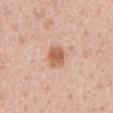Assessment:
Recorded during total-body skin imaging; not selected for excision or biopsy.
Background:
The lesion is on the abdomen. The tile uses white-light illumination. This image is a 15 mm lesion crop taken from a total-body photograph. The recorded lesion diameter is about 3 mm. A male patient, aged approximately 55. An algorithmic analysis of the crop reported a lesion area of about 5.5 mm², a shape eccentricity near 0.65, and a shape-asymmetry score of about 0.25 (0 = symmetric). And it measured a nevus-likeness score of about 95/100 and a detector confidence of about 100 out of 100 that the crop contains a lesion.